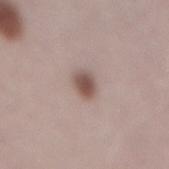The lesion was tiled from a total-body skin photograph and was not biopsied.
From the mid back.
Measured at roughly 2.5 mm in maximum diameter.
The lesion-visualizer software estimated a lesion color around L≈53 a*≈16 b*≈22 in CIELAB and a lesion-to-skin contrast of about 8.5 (normalized; higher = more distinct). And it measured border irregularity of about 1 on a 0–10 scale, internal color variation of about 4 on a 0–10 scale, and radial color variation of about 1.
A close-up tile cropped from a whole-body skin photograph, about 15 mm across.
A male patient, aged around 70.